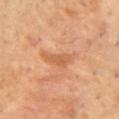Captured during whole-body skin photography for melanoma surveillance; the lesion was not biopsied. Automated tile analysis of the lesion measured a lesion area of about 4.5 mm². The analysis additionally found a mean CIELAB color near L≈59 a*≈25 b*≈39, a lesion–skin lightness drop of about 8, and a lesion-to-skin contrast of about 6 (normalized; higher = more distinct). It also reported a border-irregularity index near 4/10, a color-variation rating of about 1.5/10, and peripheral color asymmetry of about 0.5. This image is a 15 mm lesion crop taken from a total-body photograph. Located on the chest. This is a cross-polarized tile. A male subject aged 48 to 52. About 3.5 mm across.